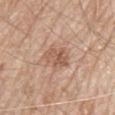The lesion-visualizer software estimated a footprint of about 8 mm², an eccentricity of roughly 0.7, and a symmetry-axis asymmetry near 0.2. And it measured roughly 9 lightness units darker than nearby skin and a normalized border contrast of about 6.5. It also reported a classifier nevus-likeness of about 5/100 and a lesion-detection confidence of about 100/100.
Approximately 3.5 mm at its widest.
Imaged with white-light lighting.
A lesion tile, about 15 mm wide, cut from a 3D total-body photograph.
Located on the mid back.
A male patient aged approximately 80.